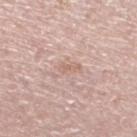- biopsy status: imaged on a skin check; not biopsied
- lesion diameter: about 2.5 mm
- patient: male, in their mid- to late 60s
- TBP lesion metrics: a footprint of about 2 mm², an eccentricity of roughly 0.95, and a shape-asymmetry score of about 0.5 (0 = symmetric)
- lighting: white-light
- location: the left lower leg
- imaging modality: 15 mm crop, total-body photography